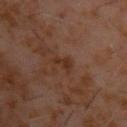Part of a total-body skin-imaging series; this lesion was reviewed on a skin check and was not flagged for biopsy.
The recorded lesion diameter is about 3 mm.
Imaged with cross-polarized lighting.
A region of skin cropped from a whole-body photographic capture, roughly 15 mm wide.
The lesion is on the upper back.
The patient is a male aged 58–62.